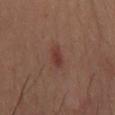No biopsy was performed on this lesion — it was imaged during a full skin examination and was not determined to be concerning. On the right thigh. The patient is a female in their 40s. Measured at roughly 3 mm in maximum diameter. A region of skin cropped from a whole-body photographic capture, roughly 15 mm wide. An algorithmic analysis of the crop reported a border-irregularity index near 3/10, internal color variation of about 0.5 on a 0–10 scale, and radial color variation of about 0. And it measured a nevus-likeness score of about 95/100 and a detector confidence of about 100 out of 100 that the crop contains a lesion.